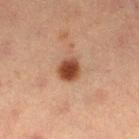follow-up=total-body-photography surveillance lesion; no biopsy
lighting=cross-polarized
lesion diameter=about 2.5 mm
image-analysis metrics=a nevus-likeness score of about 100/100
patient=female, aged approximately 55
site=the left lower leg
acquisition=total-body-photography crop, ~15 mm field of view